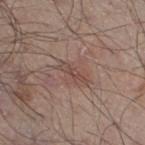<tbp_lesion>
  <biopsy_status>not biopsied; imaged during a skin examination</biopsy_status>
  <image>
    <source>total-body photography crop</source>
    <field_of_view_mm>15</field_of_view_mm>
  </image>
  <lighting>white-light</lighting>
  <lesion_size>
    <long_diameter_mm_approx>3.5</long_diameter_mm_approx>
  </lesion_size>
  <patient>
    <sex>male</sex>
    <age_approx>50</age_approx>
  </patient>
  <site>right thigh</site>
</tbp_lesion>